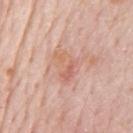Impression: Recorded during total-body skin imaging; not selected for excision or biopsy. Clinical summary: From the chest. A close-up tile cropped from a whole-body skin photograph, about 15 mm across. The patient is a male roughly 80 years of age.